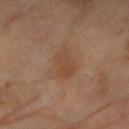  image:
    source: total-body photography crop
    field_of_view_mm: 15
  lighting: cross-polarized
  patient:
    sex: female
    age_approx: 80
  site: right forearm